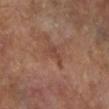notes: catalogued during a skin exam; not biopsied | tile lighting: cross-polarized | patient: female, approximately 75 years of age | lesion size: ~3 mm (longest diameter) | TBP lesion metrics: a mean CIELAB color near L≈43 a*≈21 b*≈28, roughly 6 lightness units darker than nearby skin, and a lesion-to-skin contrast of about 5.5 (normalized; higher = more distinct); border irregularity of about 4.5 on a 0–10 scale, a within-lesion color-variation index near 0/10, and radial color variation of about 0 | location: the left lower leg | acquisition: total-body-photography crop, ~15 mm field of view.